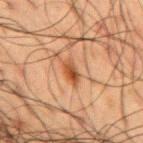  biopsy_status: not biopsied; imaged during a skin examination
  patient:
    sex: male
    age_approx: 60
  image:
    source: total-body photography crop
    field_of_view_mm: 15
  site: mid back